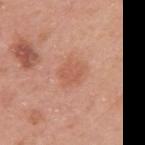| field | value |
|---|---|
| notes | total-body-photography surveillance lesion; no biopsy |
| location | the right upper arm |
| TBP lesion metrics | a lesion area of about 8 mm², an eccentricity of roughly 0.5, and two-axis asymmetry of about 0.2; a border-irregularity index near 2/10, a within-lesion color-variation index near 2/10, and a peripheral color-asymmetry measure near 0.5 |
| subject | male, aged 48–52 |
| image | 15 mm crop, total-body photography |